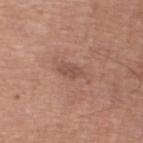The lesion was photographed on a routine skin check and not biopsied; there is no pathology result. A male patient, aged around 70. A region of skin cropped from a whole-body photographic capture, roughly 15 mm wide. From the left thigh. The recorded lesion diameter is about 3 mm.